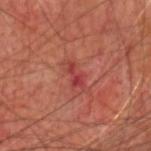{
  "biopsy_status": "not biopsied; imaged during a skin examination",
  "image": {
    "source": "total-body photography crop",
    "field_of_view_mm": 15
  },
  "patient": {
    "sex": "male",
    "age_approx": 70
  },
  "lesion_size": {
    "long_diameter_mm_approx": 3.0
  },
  "site": "head or neck",
  "lighting": "cross-polarized",
  "automated_metrics": {
    "area_mm2_approx": 3.5,
    "shape_asymmetry": 0.35
  }
}